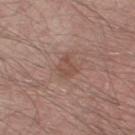workup: imaged on a skin check; not biopsied
subject: male, aged around 35
anatomic site: the right thigh
illumination: white-light
size: ~2.5 mm (longest diameter)
acquisition: ~15 mm tile from a whole-body skin photo
image-analysis metrics: a mean CIELAB color near L≈49 a*≈20 b*≈25, a lesion–skin lightness drop of about 7, and a normalized lesion–skin contrast near 5.5